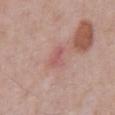workup = imaged on a skin check; not biopsied | patient = male, aged approximately 70 | body site = the chest | imaging modality = ~15 mm tile from a whole-body skin photo | diameter = about 2.5 mm | lighting = white-light.